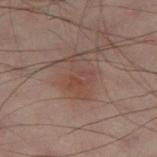Imaged during a routine full-body skin examination; the lesion was not biopsied and no histopathology is available.
Imaged with cross-polarized lighting.
The recorded lesion diameter is about 4.5 mm.
On the left thigh.
A 15 mm close-up tile from a total-body photography series done for melanoma screening.
The subject is a male in their 50s.